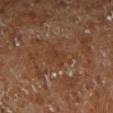biopsy status = imaged on a skin check; not biopsied
acquisition = 15 mm crop, total-body photography
anatomic site = the right lower leg
subject = male, aged 68–72
TBP lesion metrics = a shape eccentricity near 0.85 and two-axis asymmetry of about 0.5; a border-irregularity index near 5.5/10 and a peripheral color-asymmetry measure near 0.5
size = ~3 mm (longest diameter)
illumination = cross-polarized